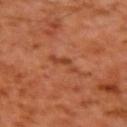  biopsy_status: not biopsied; imaged during a skin examination
  image:
    source: total-body photography crop
    field_of_view_mm: 15
  lighting: cross-polarized
  lesion_size:
    long_diameter_mm_approx: 3.0
  patient:
    sex: male
    age_approx: 60
  site: left upper arm